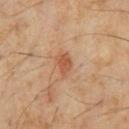Recorded during total-body skin imaging; not selected for excision or biopsy.
Located on the front of the torso.
A male patient, approximately 60 years of age.
A roughly 15 mm field-of-view crop from a total-body skin photograph.